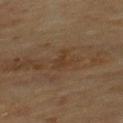The lesion is located on the mid back. The patient is a male aged 63 to 67. Captured under cross-polarized illumination. Automated image analysis of the tile measured a lesion area of about 3.5 mm², an outline eccentricity of about 0.85 (0 = round, 1 = elongated), and a shape-asymmetry score of about 0.5 (0 = symmetric). The software also gave a mean CIELAB color near L≈32 a*≈15 b*≈26, roughly 5 lightness units darker than nearby skin, and a lesion-to-skin contrast of about 5 (normalized; higher = more distinct). The analysis additionally found a border-irregularity index near 5.5/10 and a color-variation rating of about 0.5/10. And it measured a nevus-likeness score of about 0/100 and a detector confidence of about 100 out of 100 that the crop contains a lesion. A 15 mm close-up tile from a total-body photography series done for melanoma screening.